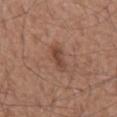workup — imaged on a skin check; not biopsied
image-analysis metrics — a lesion area of about 4.5 mm² and an outline eccentricity of about 0.9 (0 = round, 1 = elongated); a color-variation rating of about 2/10 and peripheral color asymmetry of about 0.5; a nevus-likeness score of about 25/100 and a lesion-detection confidence of about 100/100
size — about 3.5 mm
patient — male, roughly 75 years of age
location — the mid back
lighting — white-light illumination
acquisition — total-body-photography crop, ~15 mm field of view